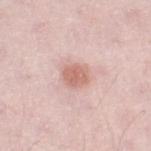biopsy_status: not biopsied; imaged during a skin examination
image:
  source: total-body photography crop
  field_of_view_mm: 15
lesion_size:
  long_diameter_mm_approx: 3.0
lighting: white-light
site: right thigh
patient:
  sex: male
  age_approx: 50